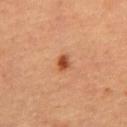Background:
A region of skin cropped from a whole-body photographic capture, roughly 15 mm wide. The lesion is located on the abdomen. A male patient in their mid-60s.